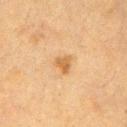Notes:
• biopsy status: imaged on a skin check; not biopsied
• acquisition: 15 mm crop, total-body photography
• lighting: cross-polarized
• diameter: about 2.5 mm
• location: the chest
• patient: female, aged approximately 55